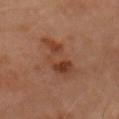Findings:
• automated metrics: a border-irregularity rating of about 5/10, internal color variation of about 5.5 on a 0–10 scale, and peripheral color asymmetry of about 1.5
• subject: female, aged around 60
• image source: total-body-photography crop, ~15 mm field of view
• lighting: cross-polarized illumination
• site: the head or neck